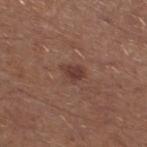| feature | finding |
|---|---|
| image | ~15 mm tile from a whole-body skin photo |
| lesion size | ≈3 mm |
| lighting | white-light |
| location | the left lower leg |
| patient | male, roughly 65 years of age |
| image-analysis metrics | a border-irregularity rating of about 2.5/10, a color-variation rating of about 2.5/10, and a peripheral color-asymmetry measure near 0.5; lesion-presence confidence of about 100/100 |